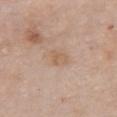This lesion was catalogued during total-body skin photography and was not selected for biopsy. A female patient, aged 63–67. Located on the chest. A roughly 15 mm field-of-view crop from a total-body skin photograph.